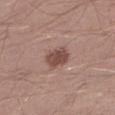notes=no biopsy performed (imaged during a skin exam); patient=male, approximately 30 years of age; imaging modality=~15 mm crop, total-body skin-cancer survey; illumination=white-light; anatomic site=the left lower leg; lesion size=~3 mm (longest diameter).